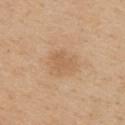Captured during whole-body skin photography for melanoma surveillance; the lesion was not biopsied.